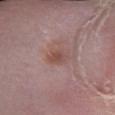This lesion was catalogued during total-body skin photography and was not selected for biopsy. Cropped from a total-body skin-imaging series; the visible field is about 15 mm. The lesion is on the right lower leg. A male patient, aged 53 to 57.